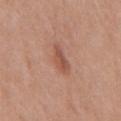<tbp_lesion>
  <biopsy_status>not biopsied; imaged during a skin examination</biopsy_status>
  <image>
    <source>total-body photography crop</source>
    <field_of_view_mm>15</field_of_view_mm>
  </image>
  <lesion_size>
    <long_diameter_mm_approx>4.0</long_diameter_mm_approx>
  </lesion_size>
  <patient>
    <sex>male</sex>
    <age_approx>70</age_approx>
  </patient>
  <automated_metrics>
    <border_irregularity_0_10>3.0</border_irregularity_0_10>
    <color_variation_0_10>3.0</color_variation_0_10>
  </automated_metrics>
  <site>chest</site>
  <lighting>white-light</lighting>
</tbp_lesion>